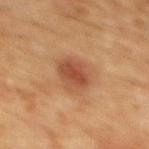* follow-up · total-body-photography surveillance lesion; no biopsy
* location · the upper back
* patient · female, aged approximately 70
* illumination · cross-polarized illumination
* image · ~15 mm crop, total-body skin-cancer survey
* lesion size · ~3.5 mm (longest diameter)